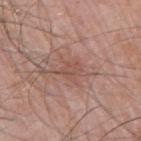notes=imaged on a skin check; not biopsied
location=the right upper arm
image source=15 mm crop, total-body photography
lesion size=about 3 mm
subject=male, in their mid-60s
lighting=white-light